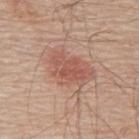- biopsy status · total-body-photography surveillance lesion; no biopsy
- tile lighting · white-light illumination
- site · the upper back
- size · about 5.5 mm
- subject · male, approximately 70 years of age
- acquisition · 15 mm crop, total-body photography